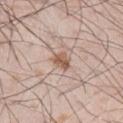biopsy status=no biopsy performed (imaged during a skin exam) | acquisition=~15 mm crop, total-body skin-cancer survey | diameter=≈2.5 mm | subject=male, in their mid-20s | tile lighting=white-light | body site=the right lower leg | TBP lesion metrics=a footprint of about 3.5 mm², an eccentricity of roughly 0.7, and a symmetry-axis asymmetry near 0.3; a classifier nevus-likeness of about 90/100 and lesion-presence confidence of about 100/100.